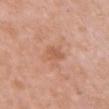image source = ~15 mm tile from a whole-body skin photo; lighting = white-light illumination; site = the chest; subject = female, aged 38–42; size = ~2.5 mm (longest diameter).